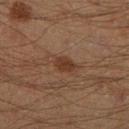No biopsy was performed on this lesion — it was imaged during a full skin examination and was not determined to be concerning. This is a cross-polarized tile. The subject is a male aged around 45. Measured at roughly 2.5 mm in maximum diameter. Located on the right lower leg. This image is a 15 mm lesion crop taken from a total-body photograph.